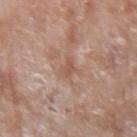This lesion was catalogued during total-body skin photography and was not selected for biopsy. The lesion is located on the right forearm. A female patient, in their mid- to late 70s. Imaged with white-light lighting. A close-up tile cropped from a whole-body skin photograph, about 15 mm across. Automated tile analysis of the lesion measured an eccentricity of roughly 0.85 and a shape-asymmetry score of about 0.35 (0 = symmetric). The analysis additionally found a border-irregularity rating of about 4/10, a within-lesion color-variation index near 0.5/10, and a peripheral color-asymmetry measure near 0. Measured at roughly 2.5 mm in maximum diameter.